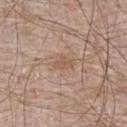{
  "biopsy_status": "not biopsied; imaged during a skin examination",
  "site": "chest",
  "image": {
    "source": "total-body photography crop",
    "field_of_view_mm": 15
  },
  "patient": {
    "sex": "male",
    "age_approx": 65
  }
}